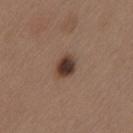Part of a total-body skin-imaging series; this lesion was reviewed on a skin check and was not flagged for biopsy.
The lesion is on the left thigh.
The tile uses white-light illumination.
A 15 mm close-up extracted from a 3D total-body photography capture.
A female subject aged 38 to 42.